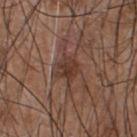This lesion was catalogued during total-body skin photography and was not selected for biopsy. Cropped from a whole-body photographic skin survey; the tile spans about 15 mm. A male subject approximately 55 years of age. Automated tile analysis of the lesion measured a lesion area of about 6.5 mm², an eccentricity of roughly 0.45, and a symmetry-axis asymmetry near 0.3. And it measured an average lesion color of about L≈36 a*≈19 b*≈25 (CIELAB), roughly 9 lightness units darker than nearby skin, and a normalized border contrast of about 7.5. The analysis additionally found border irregularity of about 3.5 on a 0–10 scale, a color-variation rating of about 3.5/10, and a peripheral color-asymmetry measure near 1. On the chest. Captured under white-light illumination. The recorded lesion diameter is about 3 mm.